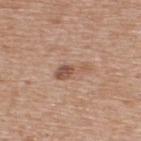Q: Was this lesion biopsied?
A: total-body-photography surveillance lesion; no biopsy
Q: Who is the patient?
A: male, aged 58–62
Q: What did automated image analysis measure?
A: a classifier nevus-likeness of about 5/100 and a detector confidence of about 100 out of 100 that the crop contains a lesion
Q: What is the anatomic site?
A: the upper back
Q: What is the imaging modality?
A: total-body-photography crop, ~15 mm field of view
Q: Lesion size?
A: ~4 mm (longest diameter)
Q: How was the tile lit?
A: white-light